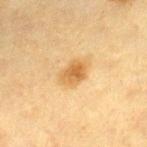follow-up — imaged on a skin check; not biopsied
imaging modality — total-body-photography crop, ~15 mm field of view
lesion diameter — ≈3.5 mm
illumination — cross-polarized illumination
body site — the leg
patient — female, aged approximately 55
automated lesion analysis — a lesion color around L≈54 a*≈17 b*≈39 in CIELAB, a lesion–skin lightness drop of about 9, and a normalized lesion–skin contrast near 7.5; a within-lesion color-variation index near 4/10 and peripheral color asymmetry of about 1; a classifier nevus-likeness of about 90/100 and a detector confidence of about 100 out of 100 that the crop contains a lesion